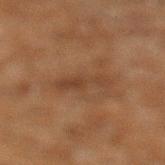The lesion was tiled from a total-body skin photograph and was not biopsied.